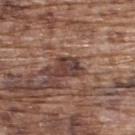– follow-up — catalogued during a skin exam; not biopsied
– tile lighting — white-light
– location — the upper back
– diameter — ~3 mm (longest diameter)
– acquisition — total-body-photography crop, ~15 mm field of view
– TBP lesion metrics — a lesion area of about 5.5 mm², a shape eccentricity near 0.6, and a shape-asymmetry score of about 0.35 (0 = symmetric); a border-irregularity index near 3.5/10 and radial color variation of about 1.5
– subject — male, in their mid- to late 70s